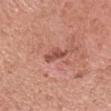Imaged during a routine full-body skin examination; the lesion was not biopsied and no histopathology is available. On the head or neck. An algorithmic analysis of the crop reported an average lesion color of about L≈51 a*≈27 b*≈28 (CIELAB), about 10 CIELAB-L* units darker than the surrounding skin, and a normalized lesion–skin contrast near 7. The patient is a female aged 53–57. The lesion's longest dimension is about 3 mm. Cropped from a whole-body photographic skin survey; the tile spans about 15 mm. The tile uses white-light illumination.